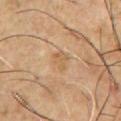Assessment:
This lesion was catalogued during total-body skin photography and was not selected for biopsy.
Context:
On the chest. The subject is a male roughly 55 years of age. A 15 mm crop from a total-body photograph taken for skin-cancer surveillance.